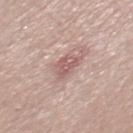Notes:
• follow-up · no biopsy performed (imaged during a skin exam)
• site · the chest
• illumination · white-light illumination
• subject · male, in their mid-50s
• automated lesion analysis · an area of roughly 6 mm², a shape eccentricity near 0.65, and a symmetry-axis asymmetry near 0.25; a lesion color around L≈58 a*≈21 b*≈21 in CIELAB, roughly 10 lightness units darker than nearby skin, and a normalized lesion–skin contrast near 6.5; a classifier nevus-likeness of about 0/100 and a lesion-detection confidence of about 100/100
• image · ~15 mm crop, total-body skin-cancer survey